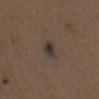This lesion was catalogued during total-body skin photography and was not selected for biopsy. Automated image analysis of the tile measured a border-irregularity index near 3/10 and internal color variation of about 4.5 on a 0–10 scale. The subject is a male in their mid-70s. The lesion is on the chest. Longest diameter approximately 3 mm. Cropped from a whole-body photographic skin survey; the tile spans about 15 mm.